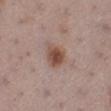{
  "biopsy_status": "not biopsied; imaged during a skin examination",
  "image": {
    "source": "total-body photography crop",
    "field_of_view_mm": 15
  },
  "patient": {
    "sex": "female",
    "age_approx": 55
  },
  "automated_metrics": {
    "nevus_likeness_0_100": 95,
    "lesion_detection_confidence_0_100": 100
  },
  "lesion_size": {
    "long_diameter_mm_approx": 3.5
  },
  "site": "left lower leg"
}